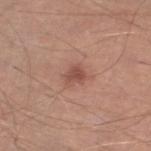{
  "biopsy_status": "not biopsied; imaged during a skin examination",
  "lighting": "white-light",
  "patient": {
    "sex": "male",
    "age_approx": 55
  },
  "image": {
    "source": "total-body photography crop",
    "field_of_view_mm": 15
  },
  "lesion_size": {
    "long_diameter_mm_approx": 2.5
  },
  "automated_metrics": {
    "nevus_likeness_0_100": 70
  },
  "site": "left lower leg"
}